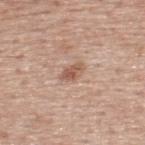Q: What did automated image analysis measure?
A: a lesion area of about 4 mm², a shape eccentricity near 0.8, and a symmetry-axis asymmetry near 0.3; an average lesion color of about L≈56 a*≈20 b*≈29 (CIELAB), a lesion–skin lightness drop of about 10, and a lesion-to-skin contrast of about 7 (normalized; higher = more distinct); a peripheral color-asymmetry measure near 1; a nevus-likeness score of about 20/100 and lesion-presence confidence of about 100/100
Q: How was the tile lit?
A: white-light illumination
Q: What is the imaging modality?
A: ~15 mm crop, total-body skin-cancer survey
Q: Lesion location?
A: the back
Q: What are the patient's age and sex?
A: male, aged approximately 75